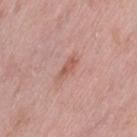lesion size: ≈3.5 mm
patient: female, approximately 40 years of age
imaging modality: ~15 mm tile from a whole-body skin photo
body site: the left thigh
image-analysis metrics: an area of roughly 4 mm², a shape eccentricity near 0.9, and a symmetry-axis asymmetry near 0.35; a lesion color around L≈57 a*≈24 b*≈27 in CIELAB and a lesion–skin lightness drop of about 8; a border-irregularity rating of about 4/10, internal color variation of about 1 on a 0–10 scale, and radial color variation of about 0; a classifier nevus-likeness of about 0/100
illumination: white-light illumination